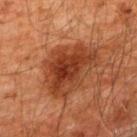follow-up — no biopsy performed (imaged during a skin exam) | location — the upper back | acquisition — ~15 mm tile from a whole-body skin photo | lesion diameter — about 6.5 mm | subject — male, in their 60s | tile lighting — cross-polarized | automated lesion analysis — a lesion color around L≈29 a*≈23 b*≈29 in CIELAB and a normalized lesion–skin contrast near 9.5; border irregularity of about 5 on a 0–10 scale, internal color variation of about 4 on a 0–10 scale, and peripheral color asymmetry of about 1.5; an automated nevus-likeness rating near 25 out of 100 and lesion-presence confidence of about 100/100.